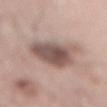<tbp_lesion>
  <biopsy_status>not biopsied; imaged during a skin examination</biopsy_status>
  <patient>
    <sex>male</sex>
    <age_approx>65</age_approx>
  </patient>
  <site>mid back</site>
  <lighting>white-light</lighting>
  <automated_metrics>
    <area_mm2_approx>18.0</area_mm2_approx>
    <eccentricity>0.75</eccentricity>
    <shape_asymmetry>0.2</shape_asymmetry>
  </automated_metrics>
  <lesion_size>
    <long_diameter_mm_approx>6.0</long_diameter_mm_approx>
  </lesion_size>
  <image>
    <source>total-body photography crop</source>
    <field_of_view_mm>15</field_of_view_mm>
  </image>
</tbp_lesion>